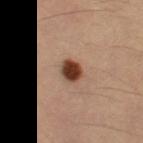The lesion was tiled from a total-body skin photograph and was not biopsied. The lesion-visualizer software estimated a lesion color around L≈41 a*≈22 b*≈29 in CIELAB and roughly 18 lightness units darker than nearby skin. The analysis additionally found a border-irregularity index near 1/10 and a color-variation rating of about 4.5/10. Cropped from a total-body skin-imaging series; the visible field is about 15 mm. This is a cross-polarized tile. A female patient aged 33–37. Measured at roughly 3 mm in maximum diameter. The lesion is on the left thigh.